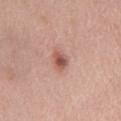  biopsy_status: not biopsied; imaged during a skin examination
  lesion_size:
    long_diameter_mm_approx: 2.5
  patient:
    sex: male
    age_approx: 80
  site: chest
  image:
    source: total-body photography crop
    field_of_view_mm: 15
  lighting: white-light